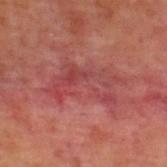| key | value |
|---|---|
| biopsy status | no biopsy performed (imaged during a skin exam) |
| tile lighting | cross-polarized |
| location | the back |
| automated lesion analysis | an area of roughly 17 mm², an outline eccentricity of about 0.85 (0 = round, 1 = elongated), and a symmetry-axis asymmetry near 0.45; an automated nevus-likeness rating near 0 out of 100 |
| subject | male, approximately 70 years of age |
| diameter | ≈7.5 mm |
| acquisition | ~15 mm tile from a whole-body skin photo |